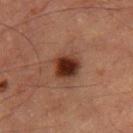Impression: Imaged during a routine full-body skin examination; the lesion was not biopsied and no histopathology is available. Acquisition and patient details: A male patient, aged 83 or older. A roughly 15 mm field-of-view crop from a total-body skin photograph. Measured at roughly 3.5 mm in maximum diameter. The lesion-visualizer software estimated a footprint of about 8.5 mm², a shape eccentricity near 0.35, and two-axis asymmetry of about 0.2. It also reported border irregularity of about 2 on a 0–10 scale and a color-variation rating of about 6/10. The software also gave an automated nevus-likeness rating near 100 out of 100 and lesion-presence confidence of about 100/100. On the right thigh. The tile uses cross-polarized illumination.